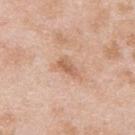Q: Was this lesion biopsied?
A: no biopsy performed (imaged during a skin exam)
Q: Where on the body is the lesion?
A: the upper back
Q: Automated lesion metrics?
A: an area of roughly 4 mm², an eccentricity of roughly 0.85, and a shape-asymmetry score of about 0.35 (0 = symmetric); roughly 10 lightness units darker than nearby skin and a normalized lesion–skin contrast near 6.5; a peripheral color-asymmetry measure near 0.5
Q: Patient demographics?
A: male, aged 23 to 27
Q: What kind of image is this?
A: ~15 mm crop, total-body skin-cancer survey
Q: What is the lesion's diameter?
A: ≈3 mm
Q: Illumination type?
A: white-light illumination